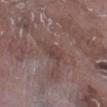Part of a total-body skin-imaging series; this lesion was reviewed on a skin check and was not flagged for biopsy. The recorded lesion diameter is about 2.5 mm. A region of skin cropped from a whole-body photographic capture, roughly 15 mm wide. Automated tile analysis of the lesion measured an average lesion color of about L≈41 a*≈17 b*≈19 (CIELAB), a lesion–skin lightness drop of about 6, and a normalized lesion–skin contrast near 5. The analysis additionally found a border-irregularity rating of about 2.5/10, internal color variation of about 2 on a 0–10 scale, and a peripheral color-asymmetry measure near 0.5. This is a white-light tile. The patient is a male aged 73–77. Located on the right lower leg.